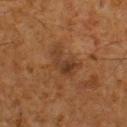Case summary:
* follow-up · catalogued during a skin exam; not biopsied
* image · ~15 mm crop, total-body skin-cancer survey
* patient · male, roughly 60 years of age
* image-analysis metrics · a mean CIELAB color near L≈31 a*≈17 b*≈27, roughly 7 lightness units darker than nearby skin, and a normalized lesion–skin contrast near 7; an automated nevus-likeness rating near 0 out of 100 and lesion-presence confidence of about 100/100
* location · the back
* illumination · cross-polarized